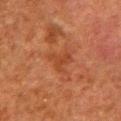  biopsy_status: not biopsied; imaged during a skin examination
  site: left lower leg
  patient:
    sex: male
    age_approx: 80
  lighting: cross-polarized
  automated_metrics:
    area_mm2_approx: 4.0
    eccentricity: 0.65
    shape_asymmetry: 0.65
    border_irregularity_0_10: 6.5
    color_variation_0_10: 1.0
    peripheral_color_asymmetry: 0.5
  image:
    source: total-body photography crop
    field_of_view_mm: 15
  lesion_size:
    long_diameter_mm_approx: 2.5